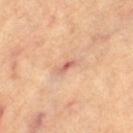Impression: Captured during whole-body skin photography for melanoma surveillance; the lesion was not biopsied. Context: A close-up tile cropped from a whole-body skin photograph, about 15 mm across. The patient is a female about 65 years old. The lesion is on the left thigh. The lesion-visualizer software estimated a shape eccentricity near 0.9 and a shape-asymmetry score of about 0.45 (0 = symmetric). This is a cross-polarized tile. Approximately 2.5 mm at its widest.